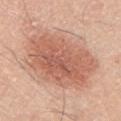Q: Was a biopsy performed?
A: no biopsy performed (imaged during a skin exam)
Q: Lesion size?
A: ≈9 mm
Q: Patient demographics?
A: male, in their mid-40s
Q: How was this image acquired?
A: 15 mm crop, total-body photography
Q: What is the anatomic site?
A: the chest